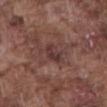Case summary:
• notes — imaged on a skin check; not biopsied
• automated lesion analysis — border irregularity of about 2.5 on a 0–10 scale, internal color variation of about 3.5 on a 0–10 scale, and a peripheral color-asymmetry measure near 1
• lesion size — ~3 mm (longest diameter)
• image source — ~15 mm tile from a whole-body skin photo
• patient — male, aged approximately 75
• body site — the front of the torso